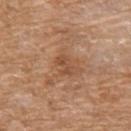No biopsy was performed on this lesion — it was imaged during a full skin examination and was not determined to be concerning.